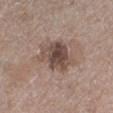Context: A female patient, aged 68–72. A 15 mm close-up tile from a total-body photography series done for melanoma screening. The recorded lesion diameter is about 5.5 mm. The lesion is on the leg. The tile uses white-light illumination. Automated tile analysis of the lesion measured a lesion area of about 15 mm². And it measured an automated nevus-likeness rating near 40 out of 100.